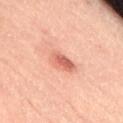The lesion was tiled from a total-body skin photograph and was not biopsied.
Imaged with cross-polarized lighting.
Cropped from a total-body skin-imaging series; the visible field is about 15 mm.
Longest diameter approximately 3.5 mm.
A male patient, aged 63–67.
The lesion is located on the right thigh.
Automated image analysis of the tile measured a border-irregularity index near 2/10, internal color variation of about 5.5 on a 0–10 scale, and radial color variation of about 2. The software also gave a nevus-likeness score of about 95/100 and a lesion-detection confidence of about 100/100.